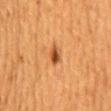Recorded during total-body skin imaging; not selected for excision or biopsy. A female patient, roughly 50 years of age. Located on the mid back. Cropped from a total-body skin-imaging series; the visible field is about 15 mm.